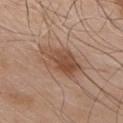This lesion was catalogued during total-body skin photography and was not selected for biopsy.
A 15 mm close-up extracted from a 3D total-body photography capture.
A male subject, in their 80s.
From the chest.